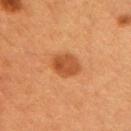Case summary:
- lesion size · ~3.5 mm (longest diameter)
- patient · female, approximately 40 years of age
- lighting · cross-polarized
- image-analysis metrics · an eccentricity of roughly 0.55 and a shape-asymmetry score of about 0.15 (0 = symmetric); a mean CIELAB color near L≈43 a*≈24 b*≈36; a nevus-likeness score of about 95/100
- site · the left upper arm
- acquisition · ~15 mm crop, total-body skin-cancer survey